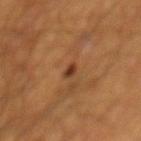Q: Who is the patient?
A: male, aged approximately 65
Q: How large is the lesion?
A: about 2 mm
Q: Lesion location?
A: the back
Q: Automated lesion metrics?
A: a footprint of about 2.5 mm² and a symmetry-axis asymmetry near 0.25; an average lesion color of about L≈34 a*≈20 b*≈30 (CIELAB), about 10 CIELAB-L* units darker than the surrounding skin, and a normalized lesion–skin contrast near 9; a classifier nevus-likeness of about 80/100 and lesion-presence confidence of about 100/100
Q: How was this image acquired?
A: 15 mm crop, total-body photography
Q: How was the tile lit?
A: cross-polarized illumination